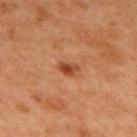Captured during whole-body skin photography for melanoma surveillance; the lesion was not biopsied. A female patient, aged 38–42. Automated tile analysis of the lesion measured a footprint of about 3.5 mm², an outline eccentricity of about 0.75 (0 = round, 1 = elongated), and a shape-asymmetry score of about 0.35 (0 = symmetric). And it measured a border-irregularity index near 2.5/10, internal color variation of about 6 on a 0–10 scale, and radial color variation of about 2. And it measured a lesion-detection confidence of about 100/100. The tile uses cross-polarized illumination. The lesion is located on the mid back. A 15 mm crop from a total-body photograph taken for skin-cancer surveillance.